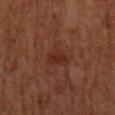Impression:
Recorded during total-body skin imaging; not selected for excision or biopsy.
Background:
A region of skin cropped from a whole-body photographic capture, roughly 15 mm wide. A male subject aged around 80. The recorded lesion diameter is about 3 mm. The lesion is located on the mid back. The total-body-photography lesion software estimated a lesion area of about 5.5 mm², an outline eccentricity of about 0.4 (0 = round, 1 = elongated), and a symmetry-axis asymmetry near 0.3. It also reported a nevus-likeness score of about 0/100. The tile uses cross-polarized illumination.